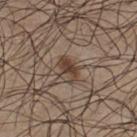Recorded during total-body skin imaging; not selected for excision or biopsy.
The patient is a male in their mid- to late 20s.
A lesion tile, about 15 mm wide, cut from a 3D total-body photograph.
The tile uses white-light illumination.
The recorded lesion diameter is about 3 mm.
Automated tile analysis of the lesion measured a border-irregularity index near 4.5/10 and a within-lesion color-variation index near 2/10.
The lesion is located on the chest.